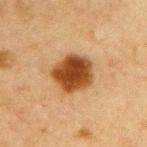  biopsy_status: not biopsied; imaged during a skin examination
  image:
    source: total-body photography crop
    field_of_view_mm: 15
  lighting: cross-polarized
  lesion_size:
    long_diameter_mm_approx: 4.5
  site: arm
  patient:
    sex: male
    age_approx: 65